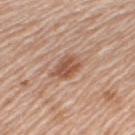Q: Was this lesion biopsied?
A: imaged on a skin check; not biopsied
Q: Lesion size?
A: ≈4 mm
Q: How was the tile lit?
A: white-light illumination
Q: What is the anatomic site?
A: the arm
Q: Who is the patient?
A: female, approximately 70 years of age
Q: Automated lesion metrics?
A: a footprint of about 7.5 mm² and a symmetry-axis asymmetry near 0.25
Q: What is the imaging modality?
A: 15 mm crop, total-body photography